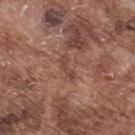The lesion was photographed on a routine skin check and not biopsied; there is no pathology result. Located on the upper back. A male subject in their mid- to late 70s. Cropped from a whole-body photographic skin survey; the tile spans about 15 mm.